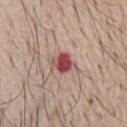| field | value |
|---|---|
| follow-up | no biopsy performed (imaged during a skin exam) |
| acquisition | total-body-photography crop, ~15 mm field of view |
| tile lighting | white-light illumination |
| lesion size | ≈3 mm |
| image-analysis metrics | about 16 CIELAB-L* units darker than the surrounding skin and a normalized border contrast of about 11; border irregularity of about 2 on a 0–10 scale and radial color variation of about 1.5; a classifier nevus-likeness of about 0/100 |
| subject | male, roughly 65 years of age |
| location | the front of the torso |